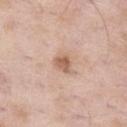Clinical impression:
No biopsy was performed on this lesion — it was imaged during a full skin examination and was not determined to be concerning.
Context:
The tile uses white-light illumination. A lesion tile, about 15 mm wide, cut from a 3D total-body photograph. The lesion-visualizer software estimated a lesion area of about 4 mm², an eccentricity of roughly 0.65, and a symmetry-axis asymmetry near 0.3. It also reported a lesion–skin lightness drop of about 11. The analysis additionally found a border-irregularity rating of about 3/10 and internal color variation of about 2.5 on a 0–10 scale. The software also gave an automated nevus-likeness rating near 55 out of 100 and a lesion-detection confidence of about 100/100. The subject is a male aged around 55. Located on the left thigh.